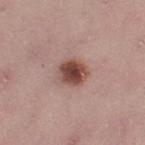- follow-up — no biopsy performed (imaged during a skin exam)
- image source — ~15 mm crop, total-body skin-cancer survey
- location — the leg
- patient — female, in their mid-20s
- tile lighting — white-light illumination